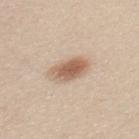Q: Was this lesion biopsied?
A: total-body-photography surveillance lesion; no biopsy
Q: Automated lesion metrics?
A: a lesion area of about 9 mm², a shape eccentricity near 0.75, and two-axis asymmetry of about 0.2; a border-irregularity rating of about 2/10, internal color variation of about 4.5 on a 0–10 scale, and radial color variation of about 1.5
Q: How was this image acquired?
A: ~15 mm tile from a whole-body skin photo
Q: What lighting was used for the tile?
A: white-light
Q: Lesion location?
A: the mid back
Q: How large is the lesion?
A: about 4 mm
Q: Who is the patient?
A: female, approximately 20 years of age